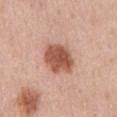Imaged during a routine full-body skin examination; the lesion was not biopsied and no histopathology is available.
A male patient aged 68 to 72.
This is a white-light tile.
A region of skin cropped from a whole-body photographic capture, roughly 15 mm wide.
About 4.5 mm across.
Automated image analysis of the tile measured a lesion-to-skin contrast of about 10 (normalized; higher = more distinct). The analysis additionally found border irregularity of about 2 on a 0–10 scale and peripheral color asymmetry of about 1.
The lesion is located on the abdomen.